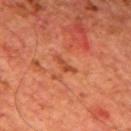Captured during whole-body skin photography for melanoma surveillance; the lesion was not biopsied. Automated tile analysis of the lesion measured a classifier nevus-likeness of about 0/100. A male patient aged 63 to 67. This is a cross-polarized tile. The lesion's longest dimension is about 3 mm. A region of skin cropped from a whole-body photographic capture, roughly 15 mm wide. From the mid back.